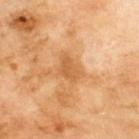Imaged during a routine full-body skin examination; the lesion was not biopsied and no histopathology is available.
The lesion is located on the upper back.
This is a cross-polarized tile.
A male subject, roughly 70 years of age.
This image is a 15 mm lesion crop taken from a total-body photograph.
Automated tile analysis of the lesion measured an average lesion color of about L≈59 a*≈24 b*≈43 (CIELAB), a lesion–skin lightness drop of about 9, and a normalized border contrast of about 6.5.